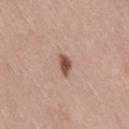<case>
  <biopsy_status>not biopsied; imaged during a skin examination</biopsy_status>
  <image>
    <source>total-body photography crop</source>
    <field_of_view_mm>15</field_of_view_mm>
  </image>
  <site>right thigh</site>
  <lighting>white-light</lighting>
  <lesion_size>
    <long_diameter_mm_approx>2.5</long_diameter_mm_approx>
  </lesion_size>
  <automated_metrics>
    <border_irregularity_0_10>2.5</border_irregularity_0_10>
    <color_variation_0_10>3.5</color_variation_0_10>
    <peripheral_color_asymmetry>1.0</peripheral_color_asymmetry>
    <nevus_likeness_0_100>100</nevus_likeness_0_100>
    <lesion_detection_confidence_0_100>100</lesion_detection_confidence_0_100>
  </automated_metrics>
  <patient>
    <sex>female</sex>
    <age_approx>40</age_approx>
  </patient>
</case>